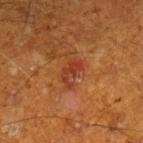<case>
<biopsy_status>not biopsied; imaged during a skin examination</biopsy_status>
<lighting>cross-polarized</lighting>
<image>
  <source>total-body photography crop</source>
  <field_of_view_mm>15</field_of_view_mm>
</image>
<patient>
  <sex>male</sex>
  <age_approx>60</age_approx>
</patient>
<site>right lower leg</site>
<lesion_size>
  <long_diameter_mm_approx>3.5</long_diameter_mm_approx>
</lesion_size>
</case>